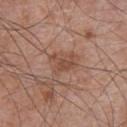Q: Was this lesion biopsied?
A: catalogued during a skin exam; not biopsied
Q: Where on the body is the lesion?
A: the abdomen
Q: What kind of image is this?
A: 15 mm crop, total-body photography
Q: What did automated image analysis measure?
A: an average lesion color of about L≈48 a*≈21 b*≈28 (CIELAB); a border-irregularity index near 4.5/10, a color-variation rating of about 2.5/10, and peripheral color asymmetry of about 1; a classifier nevus-likeness of about 0/100 and a detector confidence of about 100 out of 100 that the crop contains a lesion
Q: What lighting was used for the tile?
A: white-light
Q: Who is the patient?
A: male, about 70 years old
Q: What is the lesion's diameter?
A: ~3.5 mm (longest diameter)